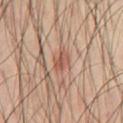Captured during whole-body skin photography for melanoma surveillance; the lesion was not biopsied. A male subject, about 60 years old. A region of skin cropped from a whole-body photographic capture, roughly 15 mm wide. The lesion is located on the chest. Imaged with cross-polarized lighting. The lesion's longest dimension is about 2.5 mm.